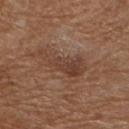Background:
This is a white-light tile. Located on the chest. The lesion-visualizer software estimated about 8 CIELAB-L* units darker than the surrounding skin and a normalized border contrast of about 6.5. A male patient, roughly 70 years of age. A 15 mm crop from a total-body photograph taken for skin-cancer surveillance. The recorded lesion diameter is about 6 mm.